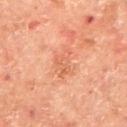Impression: Captured during whole-body skin photography for melanoma surveillance; the lesion was not biopsied. Background: The lesion is located on the upper back. The lesion-visualizer software estimated an area of roughly 5.5 mm², an outline eccentricity of about 0.7 (0 = round, 1 = elongated), and a symmetry-axis asymmetry near 0.45. The software also gave a lesion–skin lightness drop of about 7. The software also gave an automated nevus-likeness rating near 0 out of 100 and a lesion-detection confidence of about 100/100. A male subject roughly 65 years of age. Cropped from a whole-body photographic skin survey; the tile spans about 15 mm. About 3 mm across.